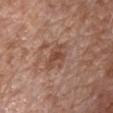  biopsy_status: not biopsied; imaged during a skin examination
  site: chest
  image:
    source: total-body photography crop
    field_of_view_mm: 15
  patient:
    sex: male
    age_approx: 80
  lesion_size:
    long_diameter_mm_approx: 4.0